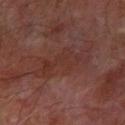<case>
<patient>
  <sex>male</sex>
  <age_approx>65</age_approx>
</patient>
<site>left forearm</site>
<image>
  <source>total-body photography crop</source>
  <field_of_view_mm>15</field_of_view_mm>
</image>
</case>